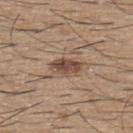Impression:
No biopsy was performed on this lesion — it was imaged during a full skin examination and was not determined to be concerning.
Background:
The total-body-photography lesion software estimated a border-irregularity index near 2/10 and a color-variation rating of about 3.5/10. And it measured a classifier nevus-likeness of about 95/100 and a lesion-detection confidence of about 100/100. The recorded lesion diameter is about 3.5 mm. The lesion is on the upper back. The patient is a male roughly 60 years of age. Cropped from a total-body skin-imaging series; the visible field is about 15 mm. This is a white-light tile.